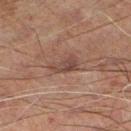Case summary:
- biopsy status: imaged on a skin check; not biopsied
- automated metrics: an area of roughly 5.5 mm², an eccentricity of roughly 0.85, and a symmetry-axis asymmetry near 0.35; a lesion color around L≈32 a*≈14 b*≈19 in CIELAB and roughly 7 lightness units darker than nearby skin; a nevus-likeness score of about 0/100 and a detector confidence of about 100 out of 100 that the crop contains a lesion
- size: ≈3.5 mm
- acquisition: 15 mm crop, total-body photography
- subject: male, aged 58–62
- anatomic site: the left lower leg
- tile lighting: cross-polarized